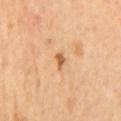This lesion was catalogued during total-body skin photography and was not selected for biopsy. From the mid back. Measured at roughly 2.5 mm in maximum diameter. A female patient approximately 55 years of age. A close-up tile cropped from a whole-body skin photograph, about 15 mm across. Automated image analysis of the tile measured a lesion area of about 2.5 mm², an outline eccentricity of about 0.85 (0 = round, 1 = elongated), and a shape-asymmetry score of about 0.3 (0 = symmetric). It also reported border irregularity of about 3 on a 0–10 scale, a color-variation rating of about 2.5/10, and a peripheral color-asymmetry measure near 1. It also reported a classifier nevus-likeness of about 85/100. This is a cross-polarized tile.